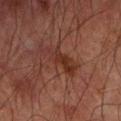Case summary:
– biopsy status — catalogued during a skin exam; not biopsied
– subject — male, aged 63–67
– automated metrics — a border-irregularity index near 6/10, a within-lesion color-variation index near 3/10, and a peripheral color-asymmetry measure near 1; a classifier nevus-likeness of about 10/100 and a lesion-detection confidence of about 95/100
– body site — the right forearm
– image — ~15 mm tile from a whole-body skin photo
– lighting — cross-polarized